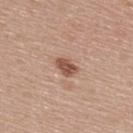follow-up=total-body-photography surveillance lesion; no biopsy | subject=female, aged approximately 60 | imaging modality=~15 mm tile from a whole-body skin photo | anatomic site=the upper back | tile lighting=white-light.